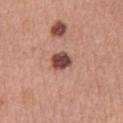| key | value |
|---|---|
| follow-up | catalogued during a skin exam; not biopsied |
| location | the right upper arm |
| subject | female, roughly 60 years of age |
| size | ≈2.5 mm |
| image | total-body-photography crop, ~15 mm field of view |
| illumination | white-light |